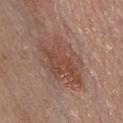biopsy status: catalogued during a skin exam; not biopsied | lesion diameter: ≈8 mm | acquisition: ~15 mm crop, total-body skin-cancer survey | subject: male, in their mid-40s | automated lesion analysis: an area of roughly 23 mm², a shape eccentricity near 0.8, and a symmetry-axis asymmetry near 0.25; a lesion color around L≈48 a*≈21 b*≈26 in CIELAB and a lesion–skin lightness drop of about 8; an automated nevus-likeness rating near 90 out of 100 and a detector confidence of about 100 out of 100 that the crop contains a lesion | lighting: white-light illumination | body site: the chest.